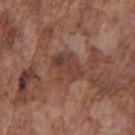Part of a total-body skin-imaging series; this lesion was reviewed on a skin check and was not flagged for biopsy.
A 15 mm crop from a total-body photograph taken for skin-cancer surveillance.
The recorded lesion diameter is about 3.5 mm.
A male patient, in their mid- to late 70s.
From the chest.
Captured under white-light illumination.